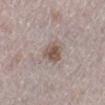On the left lower leg. A female subject in their 50s. A lesion tile, about 15 mm wide, cut from a 3D total-body photograph.